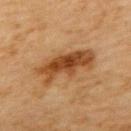<record>
  <lesion_size>
    <long_diameter_mm_approx>7.0</long_diameter_mm_approx>
  </lesion_size>
  <image>
    <source>total-body photography crop</source>
    <field_of_view_mm>15</field_of_view_mm>
  </image>
  <patient>
    <sex>female</sex>
    <age_approx>65</age_approx>
  </patient>
  <site>upper back</site>
  <lighting>cross-polarized</lighting>
  <diagnosis>
    <histopathology>dysplastic (Clark) nevus</histopathology>
    <malignancy>benign</malignancy>
    <taxonomic_path>Benign; Benign melanocytic proliferations; Nevus; Nevus, Atypical, Dysplastic, or Clark</taxonomic_path>
  </diagnosis>
</record>